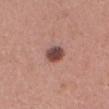Q: Was a biopsy performed?
A: imaged on a skin check; not biopsied
Q: Who is the patient?
A: female, roughly 20 years of age
Q: How was this image acquired?
A: total-body-photography crop, ~15 mm field of view
Q: Lesion location?
A: the head or neck
Q: How large is the lesion?
A: ~3 mm (longest diameter)